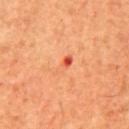body site: the mid back
size: about 3.5 mm
subject: male, roughly 60 years of age
TBP lesion metrics: an average lesion color of about L≈59 a*≈33 b*≈40 (CIELAB), roughly 8 lightness units darker than nearby skin, and a lesion-to-skin contrast of about 5.5 (normalized; higher = more distinct); a classifier nevus-likeness of about 0/100
image source: ~15 mm tile from a whole-body skin photo
lighting: cross-polarized